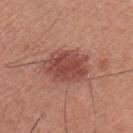Clinical impression:
This lesion was catalogued during total-body skin photography and was not selected for biopsy.
Background:
A male patient about 35 years old. On the chest. A close-up tile cropped from a whole-body skin photograph, about 15 mm across.